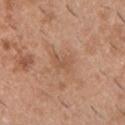Recorded during total-body skin imaging; not selected for excision or biopsy.
A 15 mm close-up tile from a total-body photography series done for melanoma screening.
A male subject, about 40 years old.
This is a white-light tile.
About 3 mm across.
Automated image analysis of the tile measured an area of roughly 5.5 mm² and an outline eccentricity of about 0.7 (0 = round, 1 = elongated).
Located on the chest.